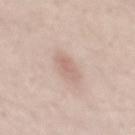Impression: The lesion was photographed on a routine skin check and not biopsied; there is no pathology result. Clinical summary: Automated image analysis of the tile measured a lesion color around L≈64 a*≈18 b*≈25 in CIELAB, about 8 CIELAB-L* units darker than the surrounding skin, and a normalized border contrast of about 5.5. The analysis additionally found an automated nevus-likeness rating near 5 out of 100 and a detector confidence of about 100 out of 100 that the crop contains a lesion. A roughly 15 mm field-of-view crop from a total-body skin photograph. The subject is a male in their mid-30s. Located on the mid back.